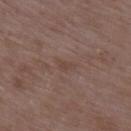Clinical summary:
A 15 mm close-up extracted from a 3D total-body photography capture. Measured at roughly 2.5 mm in maximum diameter. The lesion is on the right upper arm. The tile uses white-light illumination. The patient is a female approximately 70 years of age.